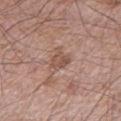Impression: Recorded during total-body skin imaging; not selected for excision or biopsy. Image and clinical context: A roughly 15 mm field-of-view crop from a total-body skin photograph. On the left lower leg. The recorded lesion diameter is about 3 mm. A male patient, aged 58 to 62.